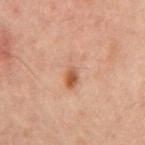Impression:
No biopsy was performed on this lesion — it was imaged during a full skin examination and was not determined to be concerning.
Image and clinical context:
On the front of the torso. A male patient aged around 60. This image is a 15 mm lesion crop taken from a total-body photograph.